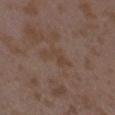Recorded during total-body skin imaging; not selected for excision or biopsy. The lesion is located on the right forearm. Cropped from a total-body skin-imaging series; the visible field is about 15 mm. A female subject about 35 years old.